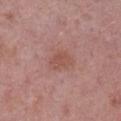Recorded during total-body skin imaging; not selected for excision or biopsy.
The subject is a female aged approximately 50.
An algorithmic analysis of the crop reported an eccentricity of roughly 0.75 and two-axis asymmetry of about 0.25. The software also gave an average lesion color of about L≈51 a*≈24 b*≈26 (CIELAB), about 7 CIELAB-L* units darker than the surrounding skin, and a normalized lesion–skin contrast near 5.5.
The lesion is located on the left lower leg.
A 15 mm crop from a total-body photograph taken for skin-cancer surveillance.